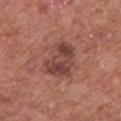biopsy_status: not biopsied; imaged during a skin examination
image:
  source: total-body photography crop
  field_of_view_mm: 15
lesion_size:
  long_diameter_mm_approx: 4.5
lighting: white-light
site: chest
patient:
  sex: male
  age_approx: 75